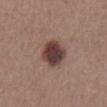Q: Was this lesion biopsied?
A: no biopsy performed (imaged during a skin exam)
Q: Automated lesion metrics?
A: a classifier nevus-likeness of about 75/100
Q: Where on the body is the lesion?
A: the abdomen
Q: What is the imaging modality?
A: ~15 mm tile from a whole-body skin photo
Q: Who is the patient?
A: male, aged 38 to 42
Q: Lesion size?
A: about 3.5 mm
Q: Illumination type?
A: white-light illumination